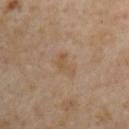Impression:
The lesion was photographed on a routine skin check and not biopsied; there is no pathology result.
Background:
The lesion's longest dimension is about 3 mm. A 15 mm close-up extracted from a 3D total-body photography capture. A male patient, about 55 years old. The lesion-visualizer software estimated an eccentricity of roughly 0.9 and a symmetry-axis asymmetry near 0.55. The analysis additionally found an average lesion color of about L≈52 a*≈16 b*≈32 (CIELAB), about 6 CIELAB-L* units darker than the surrounding skin, and a normalized border contrast of about 5.5. This is a cross-polarized tile. From the right upper arm.